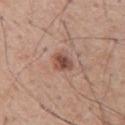Q: Was this lesion biopsied?
A: no biopsy performed (imaged during a skin exam)
Q: What kind of image is this?
A: 15 mm crop, total-body photography
Q: What lighting was used for the tile?
A: white-light illumination
Q: How large is the lesion?
A: ~2.5 mm (longest diameter)
Q: Where on the body is the lesion?
A: the upper back
Q: What are the patient's age and sex?
A: male, about 65 years old